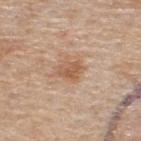Clinical impression:
This lesion was catalogued during total-body skin photography and was not selected for biopsy.
Acquisition and patient details:
The patient is a male aged 53 to 57. Imaged with white-light lighting. An algorithmic analysis of the crop reported border irregularity of about 2.5 on a 0–10 scale and a peripheral color-asymmetry measure near 1. And it measured a classifier nevus-likeness of about 50/100. The lesion is located on the upper back. Longest diameter approximately 2.5 mm. A 15 mm close-up extracted from a 3D total-body photography capture.